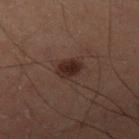<case>
<biopsy_status>not biopsied; imaged during a skin examination</biopsy_status>
<site>right thigh</site>
<automated_metrics>
  <area_mm2_approx>5.0</area_mm2_approx>
  <eccentricity>0.55</eccentricity>
  <shape_asymmetry>0.2</shape_asymmetry>
  <cielab_L>18</cielab_L>
  <cielab_a>13</cielab_a>
  <cielab_b>16</cielab_b>
  <vs_skin_darker_L>8.0</vs_skin_darker_L>
  <nevus_likeness_0_100>90</nevus_likeness_0_100>
  <lesion_detection_confidence_0_100>100</lesion_detection_confidence_0_100>
</automated_metrics>
<image>
  <source>total-body photography crop</source>
  <field_of_view_mm>15</field_of_view_mm>
</image>
<patient>
  <sex>male</sex>
  <age_approx>60</age_approx>
</patient>
</case>